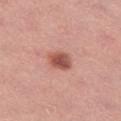Assessment:
Captured during whole-body skin photography for melanoma surveillance; the lesion was not biopsied.
Background:
A female subject, aged around 45. Captured under white-light illumination. A 15 mm close-up tile from a total-body photography series done for melanoma screening. Located on the left thigh.